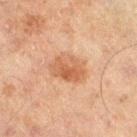No biopsy was performed on this lesion — it was imaged during a full skin examination and was not determined to be concerning. Longest diameter approximately 4.5 mm. The subject is a male in their 70s. A lesion tile, about 15 mm wide, cut from a 3D total-body photograph. This is a cross-polarized tile. On the right thigh.